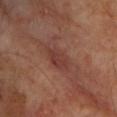follow-up = no biopsy performed (imaged during a skin exam) | patient = male, in their mid-60s | acquisition = 15 mm crop, total-body photography | illumination = cross-polarized illumination | body site = the chest.